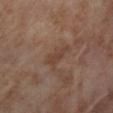{"biopsy_status": "not biopsied; imaged during a skin examination", "lesion_size": {"long_diameter_mm_approx": 3.5}, "lighting": "cross-polarized", "patient": {"sex": "female", "age_approx": 55}, "automated_metrics": {"cielab_L": 41, "cielab_a": 18, "cielab_b": 26, "vs_skin_darker_L": 6.0, "vs_skin_contrast_norm": 5.5, "border_irregularity_0_10": 3.5, "color_variation_0_10": 1.0}, "site": "right lower leg", "image": {"source": "total-body photography crop", "field_of_view_mm": 15}}